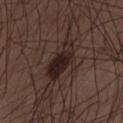Captured during whole-body skin photography for melanoma surveillance; the lesion was not biopsied. The subject is a male aged around 50. Automated image analysis of the tile measured a border-irregularity rating of about 5/10, a color-variation rating of about 5.5/10, and a peripheral color-asymmetry measure near 2. Located on the left thigh. This is a white-light tile. A roughly 15 mm field-of-view crop from a total-body skin photograph. The recorded lesion diameter is about 5.5 mm.